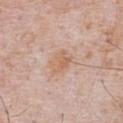From the chest. A lesion tile, about 15 mm wide, cut from a 3D total-body photograph. The patient is a male approximately 70 years of age.